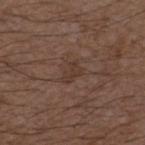The subject is a male aged 48–52.
A 15 mm crop from a total-body photograph taken for skin-cancer surveillance.
From the chest.